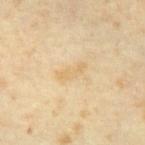Imaged during a routine full-body skin examination; the lesion was not biopsied and no histopathology is available. A male patient, aged 63–67. A 15 mm close-up extracted from a 3D total-body photography capture. About 3.5 mm across. This is a cross-polarized tile.